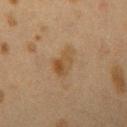* follow-up — imaged on a skin check; not biopsied
* automated metrics — an outline eccentricity of about 0.75 (0 = round, 1 = elongated); a color-variation rating of about 5/10 and peripheral color asymmetry of about 1.5; a classifier nevus-likeness of about 15/100 and a lesion-detection confidence of about 100/100
* lesion diameter — ≈3.5 mm
* anatomic site — the right upper arm
* lighting — cross-polarized
* acquisition — ~15 mm crop, total-body skin-cancer survey
* subject — female, about 40 years old